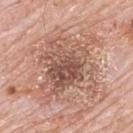Q: Was a biopsy performed?
A: no biopsy performed (imaged during a skin exam)
Q: What did automated image analysis measure?
A: a lesion color around L≈56 a*≈21 b*≈27 in CIELAB, a lesion–skin lightness drop of about 10, and a normalized lesion–skin contrast near 7; a border-irregularity index near 8/10, a color-variation rating of about 8/10, and radial color variation of about 2.5; a classifier nevus-likeness of about 0/100 and a detector confidence of about 100 out of 100 that the crop contains a lesion
Q: What is the anatomic site?
A: the upper back
Q: Who is the patient?
A: male, aged around 80
Q: How was this image acquired?
A: ~15 mm crop, total-body skin-cancer survey